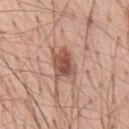Assessment: No biopsy was performed on this lesion — it was imaged during a full skin examination and was not determined to be concerning.